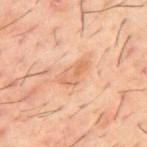The lesion was tiled from a total-body skin photograph and was not biopsied. An algorithmic analysis of the crop reported a lesion area of about 6 mm² and an outline eccentricity of about 0.9 (0 = round, 1 = elongated). It also reported a within-lesion color-variation index near 4/10 and a peripheral color-asymmetry measure near 1.5. From the mid back. A 15 mm close-up extracted from a 3D total-body photography capture. Approximately 4 mm at its widest. A male patient aged 58 to 62.